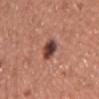{"biopsy_status": "not biopsied; imaged during a skin examination", "patient": {"sex": "male", "age_approx": 45}, "lighting": "white-light", "site": "chest", "image": {"source": "total-body photography crop", "field_of_view_mm": 15}}